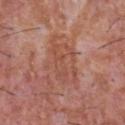workup: no biopsy performed (imaged during a skin exam)
body site: the chest
acquisition: total-body-photography crop, ~15 mm field of view
subject: male, aged 43 to 47
lighting: white-light illumination
diameter: about 6 mm
automated metrics: a footprint of about 16 mm²; a lesion-to-skin contrast of about 5 (normalized; higher = more distinct); a border-irregularity index near 4/10, a color-variation rating of about 4/10, and a peripheral color-asymmetry measure near 1; lesion-presence confidence of about 90/100